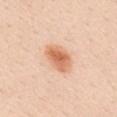Clinical impression: Imaged during a routine full-body skin examination; the lesion was not biopsied and no histopathology is available. Clinical summary: The tile uses white-light illumination. The total-body-photography lesion software estimated a lesion area of about 9 mm², a shape eccentricity near 0.75, and a shape-asymmetry score of about 0.15 (0 = symmetric). And it measured roughly 13 lightness units darker than nearby skin and a normalized lesion–skin contrast near 8.5. The software also gave a border-irregularity rating of about 1.5/10, a color-variation rating of about 5.5/10, and a peripheral color-asymmetry measure near 1.5. A 15 mm crop from a total-body photograph taken for skin-cancer surveillance. A female subject, in their mid-40s. Located on the back. Measured at roughly 4 mm in maximum diameter.